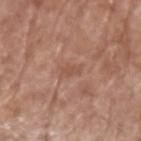Imaged during a routine full-body skin examination; the lesion was not biopsied and no histopathology is available.
The lesion is located on the left upper arm.
A 15 mm close-up tile from a total-body photography series done for melanoma screening.
This is a white-light tile.
The patient is a male roughly 75 years of age.
The recorded lesion diameter is about 2.5 mm.